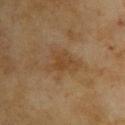This is a cross-polarized tile. Located on the back. A female patient, approximately 60 years of age. A 15 mm crop from a total-body photograph taken for skin-cancer surveillance.